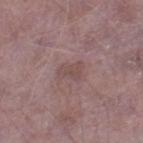This lesion was catalogued during total-body skin photography and was not selected for biopsy.
The total-body-photography lesion software estimated a footprint of about 3 mm² and a shape eccentricity near 0.75. And it measured border irregularity of about 5 on a 0–10 scale and radial color variation of about 0.5. It also reported an automated nevus-likeness rating near 0 out of 100 and a detector confidence of about 100 out of 100 that the crop contains a lesion.
The lesion is on the right lower leg.
A male subject, in their mid-60s.
A lesion tile, about 15 mm wide, cut from a 3D total-body photograph.
Longest diameter approximately 2.5 mm.
This is a white-light tile.